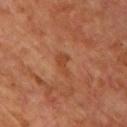Captured during whole-body skin photography for melanoma surveillance; the lesion was not biopsied. This is a cross-polarized tile. A male patient, aged approximately 65. A lesion tile, about 15 mm wide, cut from a 3D total-body photograph. Longest diameter approximately 3 mm. Located on the chest.